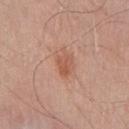Imaged during a routine full-body skin examination; the lesion was not biopsied and no histopathology is available.
The lesion-visualizer software estimated a lesion color around L≈55 a*≈24 b*≈31 in CIELAB and roughly 8 lightness units darker than nearby skin. The analysis additionally found a within-lesion color-variation index near 3.5/10 and peripheral color asymmetry of about 1.5.
The lesion is on the chest.
The subject is a male roughly 80 years of age.
Imaged with white-light lighting.
A 15 mm close-up tile from a total-body photography series done for melanoma screening.
Measured at roughly 2.5 mm in maximum diameter.